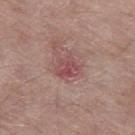Q: Is there a histopathology result?
A: imaged on a skin check; not biopsied
Q: Who is the patient?
A: male, aged around 65
Q: How was this image acquired?
A: 15 mm crop, total-body photography
Q: Where on the body is the lesion?
A: the left thigh